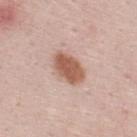notes: catalogued during a skin exam; not biopsied
location: the upper back
tile lighting: white-light illumination
image-analysis metrics: a lesion area of about 10 mm², an outline eccentricity of about 0.8 (0 = round, 1 = elongated), and a symmetry-axis asymmetry near 0.2; a mean CIELAB color near L≈58 a*≈21 b*≈29, roughly 14 lightness units darker than nearby skin, and a lesion-to-skin contrast of about 9.5 (normalized; higher = more distinct); a border-irregularity index near 2/10, a within-lesion color-variation index near 3/10, and radial color variation of about 1; a nevus-likeness score of about 95/100
lesion diameter: ~4.5 mm (longest diameter)
patient: male, aged approximately 25
imaging modality: 15 mm crop, total-body photography